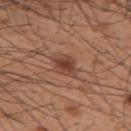Imaged during a routine full-body skin examination; the lesion was not biopsied and no histopathology is available.
Captured under white-light illumination.
The lesion is on the back.
The lesion's longest dimension is about 3.5 mm.
A 15 mm crop from a total-body photograph taken for skin-cancer surveillance.
An algorithmic analysis of the crop reported a lesion area of about 6 mm², a shape eccentricity near 0.75, and two-axis asymmetry of about 0.25. And it measured an average lesion color of about L≈43 a*≈23 b*≈27 (CIELAB), about 10 CIELAB-L* units darker than the surrounding skin, and a normalized lesion–skin contrast near 8.5. And it measured border irregularity of about 3 on a 0–10 scale, a color-variation rating of about 3.5/10, and a peripheral color-asymmetry measure near 1.
A male patient in their 60s.